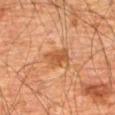Captured during whole-body skin photography for melanoma surveillance; the lesion was not biopsied. Measured at roughly 3 mm in maximum diameter. Captured under cross-polarized illumination. A male subject, approximately 80 years of age. Located on the abdomen. A roughly 15 mm field-of-view crop from a total-body skin photograph. The lesion-visualizer software estimated a footprint of about 6 mm², an eccentricity of roughly 0.7, and a symmetry-axis asymmetry near 0.25. And it measured border irregularity of about 3 on a 0–10 scale and radial color variation of about 1.